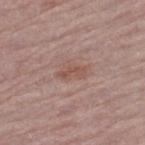The lesion was photographed on a routine skin check and not biopsied; there is no pathology result. Located on the leg. A male patient aged approximately 75. An algorithmic analysis of the crop reported a mean CIELAB color near L≈52 a*≈20 b*≈25 and a lesion–skin lightness drop of about 7. The analysis additionally found a color-variation rating of about 2/10 and a peripheral color-asymmetry measure near 0.5. A close-up tile cropped from a whole-body skin photograph, about 15 mm across. This is a white-light tile.